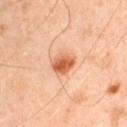workup: total-body-photography surveillance lesion; no biopsy
illumination: cross-polarized
patient: male, aged approximately 45
image: ~15 mm tile from a whole-body skin photo
anatomic site: the left upper arm
lesion size: about 3 mm